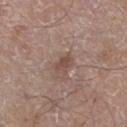The lesion was tiled from a total-body skin photograph and was not biopsied. On the leg. This image is a 15 mm lesion crop taken from a total-body photograph. A male subject, roughly 60 years of age. This is a white-light tile. Approximately 2.5 mm at its widest.